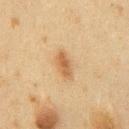Image and clinical context: The lesion is on the chest. A male patient about 60 years old. A 15 mm crop from a total-body photograph taken for skin-cancer surveillance. The recorded lesion diameter is about 4 mm. Imaged with cross-polarized lighting.